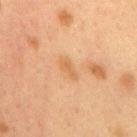{"biopsy_status": "not biopsied; imaged during a skin examination", "patient": {"sex": "female", "age_approx": 40}, "image": {"source": "total-body photography crop", "field_of_view_mm": 15}, "site": "mid back"}